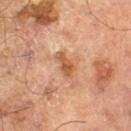Clinical impression:
The lesion was photographed on a routine skin check and not biopsied; there is no pathology result.
Clinical summary:
Automated image analysis of the tile measured a footprint of about 4.5 mm² and an eccentricity of roughly 0.85. The lesion is located on the leg. A 15 mm crop from a total-body photograph taken for skin-cancer surveillance. A male patient, aged 68–72.